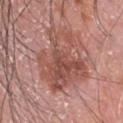Part of a total-body skin-imaging series; this lesion was reviewed on a skin check and was not flagged for biopsy.